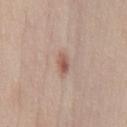notes: total-body-photography surveillance lesion; no biopsy
diameter: ~2.5 mm (longest diameter)
site: the chest
TBP lesion metrics: an average lesion color of about L≈56 a*≈20 b*≈26 (CIELAB), a lesion–skin lightness drop of about 10, and a normalized lesion–skin contrast near 7; a classifier nevus-likeness of about 65/100 and lesion-presence confidence of about 100/100
lighting: white-light
patient: male, roughly 45 years of age
acquisition: total-body-photography crop, ~15 mm field of view